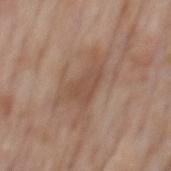Recorded during total-body skin imaging; not selected for excision or biopsy.
A male patient aged 73–77.
The lesion is located on the mid back.
Cropped from a whole-body photographic skin survey; the tile spans about 15 mm.
Longest diameter approximately 4.5 mm.